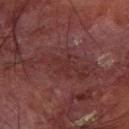No biopsy was performed on this lesion — it was imaged during a full skin examination and was not determined to be concerning. Automated tile analysis of the lesion measured a footprint of about 4.5 mm², a shape eccentricity near 0.5, and a shape-asymmetry score of about 0.5 (0 = symmetric). And it measured a mean CIELAB color near L≈24 a*≈20 b*≈17, roughly 4 lightness units darker than nearby skin, and a normalized border contrast of about 4.5. The analysis additionally found a within-lesion color-variation index near 0.5/10. A roughly 15 mm field-of-view crop from a total-body skin photograph. On the right forearm. A male patient in their 70s. The tile uses cross-polarized illumination.